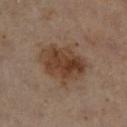subject — female, about 60 years old
imaging modality — total-body-photography crop, ~15 mm field of view
automated metrics — an area of roughly 21 mm² and an eccentricity of roughly 0.65; an average lesion color of about L≈40 a*≈17 b*≈28 (CIELAB), roughly 10 lightness units darker than nearby skin, and a lesion-to-skin contrast of about 9 (normalized; higher = more distinct)
lighting — cross-polarized
body site — the right lower leg
lesion size — ≈6 mm